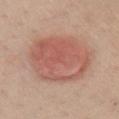biopsy status: imaged on a skin check; not biopsied
imaging modality: ~15 mm crop, total-body skin-cancer survey
diameter: about 8.5 mm
lighting: white-light illumination
site: the chest
automated lesion analysis: an automated nevus-likeness rating near 90 out of 100 and a lesion-detection confidence of about 100/100
patient: female, approximately 50 years of age